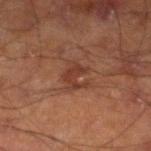| field | value |
|---|---|
| workup | no biopsy performed (imaged during a skin exam) |
| lesion diameter | ≈3 mm |
| subject | male, aged 68 to 72 |
| site | the leg |
| TBP lesion metrics | border irregularity of about 6.5 on a 0–10 scale |
| image source | 15 mm crop, total-body photography |
| tile lighting | cross-polarized illumination |